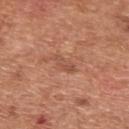notes=total-body-photography surveillance lesion; no biopsy | subject=male, aged approximately 65 | imaging modality=~15 mm tile from a whole-body skin photo | location=the back.